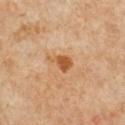<lesion>
<biopsy_status>not biopsied; imaged during a skin examination</biopsy_status>
<image>
  <source>total-body photography crop</source>
  <field_of_view_mm>15</field_of_view_mm>
</image>
<lesion_size>
  <long_diameter_mm_approx>3.0</long_diameter_mm_approx>
</lesion_size>
<patient>
  <sex>female</sex>
  <age_approx>50</age_approx>
</patient>
<site>right lower leg</site>
<lighting>cross-polarized</lighting>
</lesion>